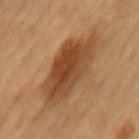Q: Was this lesion biopsied?
A: catalogued during a skin exam; not biopsied